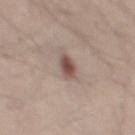Imaged during a routine full-body skin examination; the lesion was not biopsied and no histopathology is available.
A male subject in their mid-30s.
The lesion-visualizer software estimated a lesion area of about 4 mm² and an eccentricity of roughly 0.65. The analysis additionally found a lesion color around L≈50 a*≈16 b*≈23 in CIELAB, roughly 13 lightness units darker than nearby skin, and a normalized lesion–skin contrast near 9.
A 15 mm close-up extracted from a 3D total-body photography capture.
The lesion is on the mid back.
This is a white-light tile.
The lesion's longest dimension is about 2.5 mm.